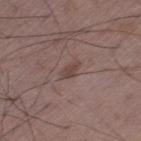This lesion was catalogued during total-body skin photography and was not selected for biopsy.
A 15 mm close-up tile from a total-body photography series done for melanoma screening.
Measured at roughly 2.5 mm in maximum diameter.
The patient is a male about 50 years old.
Located on the left thigh.
Imaged with white-light lighting.